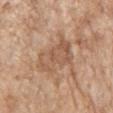site: upper back
lighting: white-light
patient:
  sex: male
  age_approx: 85+
image:
  source: total-body photography crop
  field_of_view_mm: 15
lesion_size:
  long_diameter_mm_approx: 5.0